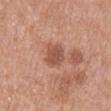Imaged with white-light lighting. Located on the back. The lesion-visualizer software estimated a mean CIELAB color near L≈52 a*≈24 b*≈29, roughly 11 lightness units darker than nearby skin, and a lesion-to-skin contrast of about 7.5 (normalized; higher = more distinct). A 15 mm close-up tile from a total-body photography series done for melanoma screening. Longest diameter approximately 3.5 mm. A male subject, aged 68–72.